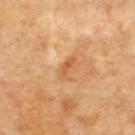follow-up — total-body-photography surveillance lesion; no biopsy
image-analysis metrics — an area of roughly 3.5 mm² and a shape-asymmetry score of about 0.3 (0 = symmetric)
anatomic site — the upper back
illumination — cross-polarized
subject — aged around 60
image source — total-body-photography crop, ~15 mm field of view
lesion diameter — about 3 mm